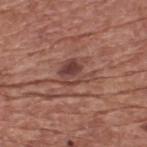workup — no biopsy performed (imaged during a skin exam); patient — male, in their mid-70s; image source — ~15 mm crop, total-body skin-cancer survey; anatomic site — the upper back.